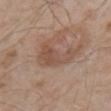Clinical impression: This lesion was catalogued during total-body skin photography and was not selected for biopsy. Acquisition and patient details: The subject is a male in their mid- to late 50s. A 15 mm crop from a total-body photograph taken for skin-cancer surveillance. The total-body-photography lesion software estimated an area of roughly 11 mm², an outline eccentricity of about 0.8 (0 = round, 1 = elongated), and two-axis asymmetry of about 0.55. It also reported about 8 CIELAB-L* units darker than the surrounding skin and a normalized lesion–skin contrast near 6. The software also gave a border-irregularity index near 7/10 and peripheral color asymmetry of about 1. The analysis additionally found a nevus-likeness score of about 25/100 and a lesion-detection confidence of about 100/100. On the right upper arm. Captured under white-light illumination. The lesion's longest dimension is about 5 mm.